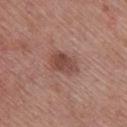lesion diameter: ~4 mm (longest diameter) | subject: female, in their mid- to late 60s | lighting: white-light | imaging modality: 15 mm crop, total-body photography | anatomic site: the front of the torso.